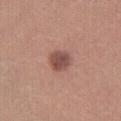Notes:
– follow-up: total-body-photography surveillance lesion; no biopsy
– image: ~15 mm crop, total-body skin-cancer survey
– lesion diameter: ~3 mm (longest diameter)
– subject: female, roughly 20 years of age
– lighting: white-light
– location: the leg